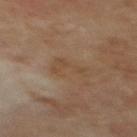Imaged during a routine full-body skin examination; the lesion was not biopsied and no histopathology is available. The patient is a male aged 68 to 72. This is a cross-polarized tile. The lesion is on the mid back. This image is a 15 mm lesion crop taken from a total-body photograph. An algorithmic analysis of the crop reported a lesion area of about 5 mm², a shape eccentricity near 0.85, and two-axis asymmetry of about 0.55.